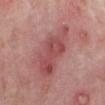<lesion>
  <biopsy_status>not biopsied; imaged during a skin examination</biopsy_status>
  <site>right forearm</site>
  <lesion_size>
    <long_diameter_mm_approx>7.5</long_diameter_mm_approx>
  </lesion_size>
  <image>
    <source>total-body photography crop</source>
    <field_of_view_mm>15</field_of_view_mm>
  </image>
  <patient>
    <sex>male</sex>
    <age_approx>75</age_approx>
  </patient>
</lesion>